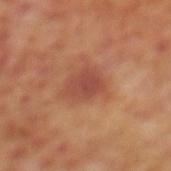biopsy status = total-body-photography surveillance lesion; no biopsy | image = ~15 mm tile from a whole-body skin photo | subject = male, aged 68 to 72 | lighting = cross-polarized illumination | automated metrics = a nevus-likeness score of about 35/100 and a detector confidence of about 100 out of 100 that the crop contains a lesion | lesion diameter = about 3.5 mm | site = the mid back.